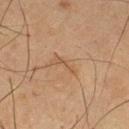Impression:
Imaged during a routine full-body skin examination; the lesion was not biopsied and no histopathology is available.
Background:
From the left thigh. A male subject, approximately 65 years of age. An algorithmic analysis of the crop reported a nevus-likeness score of about 0/100 and a lesion-detection confidence of about 100/100. Approximately 3 mm at its widest. Captured under cross-polarized illumination. A region of skin cropped from a whole-body photographic capture, roughly 15 mm wide.